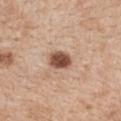<lesion>
<biopsy_status>not biopsied; imaged during a skin examination</biopsy_status>
<site>mid back</site>
<image>
  <source>total-body photography crop</source>
  <field_of_view_mm>15</field_of_view_mm>
</image>
<patient>
  <sex>female</sex>
  <age_approx>40</age_approx>
</patient>
</lesion>